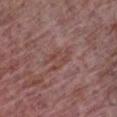Clinical impression:
The lesion was tiled from a total-body skin photograph and was not biopsied.
Clinical summary:
The lesion is located on the chest. A region of skin cropped from a whole-body photographic capture, roughly 15 mm wide. A male patient, approximately 65 years of age.